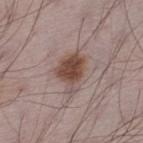Recorded during total-body skin imaging; not selected for excision or biopsy.